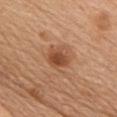notes = no biopsy performed (imaged during a skin exam)
site = the chest
automated metrics = a lesion color around L≈49 a*≈24 b*≈35 in CIELAB and about 11 CIELAB-L* units darker than the surrounding skin; a color-variation rating of about 3.5/10 and radial color variation of about 1; a classifier nevus-likeness of about 90/100 and lesion-presence confidence of about 100/100
patient = female, roughly 60 years of age
acquisition = 15 mm crop, total-body photography